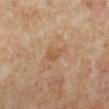The lesion was photographed on a routine skin check and not biopsied; there is no pathology result. An algorithmic analysis of the crop reported a mean CIELAB color near L≈55 a*≈19 b*≈34 and about 7 CIELAB-L* units darker than the surrounding skin. The software also gave a border-irregularity index near 3.5/10, internal color variation of about 1.5 on a 0–10 scale, and radial color variation of about 0.5. On the left lower leg. Imaged with cross-polarized lighting. The patient is a male about 65 years old. A region of skin cropped from a whole-body photographic capture, roughly 15 mm wide.